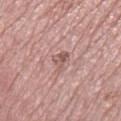workup=total-body-photography surveillance lesion; no biopsy
body site=the right thigh
subject=female, roughly 70 years of age
acquisition=total-body-photography crop, ~15 mm field of view
automated lesion analysis=an eccentricity of roughly 0.65 and two-axis asymmetry of about 0.45; a border-irregularity rating of about 4.5/10, internal color variation of about 4.5 on a 0–10 scale, and a peripheral color-asymmetry measure near 2
illumination=white-light illumination